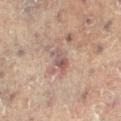The lesion was tiled from a total-body skin photograph and was not biopsied. An algorithmic analysis of the crop reported a lesion area of about 4 mm², an outline eccentricity of about 0.85 (0 = round, 1 = elongated), and two-axis asymmetry of about 0.35. The analysis additionally found a mean CIELAB color near L≈49 a*≈17 b*≈20 and a lesion-to-skin contrast of about 7.5 (normalized; higher = more distinct). It also reported a classifier nevus-likeness of about 0/100 and a detector confidence of about 90 out of 100 that the crop contains a lesion. Longest diameter approximately 3 mm. A female patient, about 80 years old. A region of skin cropped from a whole-body photographic capture, roughly 15 mm wide. The lesion is on the right leg. This is a cross-polarized tile.